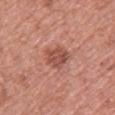workup: no biopsy performed (imaged during a skin exam) | tile lighting: white-light | image: 15 mm crop, total-body photography | body site: the front of the torso | subject: female, approximately 60 years of age | lesion diameter: about 3.5 mm | TBP lesion metrics: a footprint of about 7 mm² and an outline eccentricity of about 0.7 (0 = round, 1 = elongated); a border-irregularity index near 2.5/10, internal color variation of about 3 on a 0–10 scale, and peripheral color asymmetry of about 1; a classifier nevus-likeness of about 40/100 and lesion-presence confidence of about 100/100.